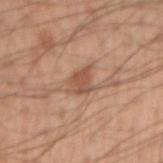  biopsy_status: not biopsied; imaged during a skin examination
  site: right upper arm
  lighting: white-light
  patient:
    sex: male
    age_approx: 55
  image:
    source: total-body photography crop
    field_of_view_mm: 15